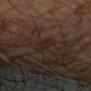Assessment:
Captured during whole-body skin photography for melanoma surveillance; the lesion was not biopsied.
Context:
About 3 mm across. On the right forearm. The patient is a male aged around 65. A close-up tile cropped from a whole-body skin photograph, about 15 mm across. The lesion-visualizer software estimated an outline eccentricity of about 0.9 (0 = round, 1 = elongated). The software also gave a nevus-likeness score of about 0/100. Imaged with cross-polarized lighting.